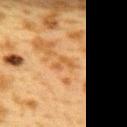The patient is a female aged 38 to 42.
Located on the upper back.
A 15 mm close-up extracted from a 3D total-body photography capture.